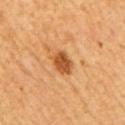The lesion was tiled from a total-body skin photograph and was not biopsied.
A male subject aged 58 to 62.
A lesion tile, about 15 mm wide, cut from a 3D total-body photograph.
From the right upper arm.
An algorithmic analysis of the crop reported a lesion area of about 6 mm² and an eccentricity of roughly 0.65. And it measured an average lesion color of about L≈52 a*≈26 b*≈43 (CIELAB), roughly 13 lightness units darker than nearby skin, and a normalized lesion–skin contrast near 9. It also reported a border-irregularity rating of about 1.5/10 and a peripheral color-asymmetry measure near 1.
Measured at roughly 3 mm in maximum diameter.